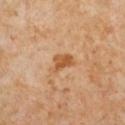<tbp_lesion>
<biopsy_status>not biopsied; imaged during a skin examination</biopsy_status>
<site>right lower leg</site>
<image>
  <source>total-body photography crop</source>
  <field_of_view_mm>15</field_of_view_mm>
</image>
<patient>
  <sex>female</sex>
  <age_approx>50</age_approx>
</patient>
</tbp_lesion>